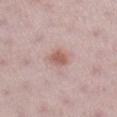notes = imaged on a skin check; not biopsied | image source = ~15 mm crop, total-body skin-cancer survey | body site = the right lower leg | lesion diameter = ≈2.5 mm | subject = female, aged approximately 30 | tile lighting = white-light illumination.